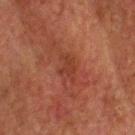<tbp_lesion>
  <biopsy_status>not biopsied; imaged during a skin examination</biopsy_status>
  <lighting>cross-polarized</lighting>
  <patient>
    <sex>male</sex>
    <age_approx>60</age_approx>
  </patient>
  <lesion_size>
    <long_diameter_mm_approx>3.5</long_diameter_mm_approx>
  </lesion_size>
  <automated_metrics>
    <area_mm2_approx>4.5</area_mm2_approx>
    <vs_skin_darker_L>5.0</vs_skin_darker_L>
    <vs_skin_contrast_norm>5.5</vs_skin_contrast_norm>
    <border_irregularity_0_10>5.5</border_irregularity_0_10>
    <color_variation_0_10>1.0</color_variation_0_10>
    <peripheral_color_asymmetry>0.5</peripheral_color_asymmetry>
    <nevus_likeness_0_100>0</nevus_likeness_0_100>
    <lesion_detection_confidence_0_100>100</lesion_detection_confidence_0_100>
  </automated_metrics>
  <image>
    <source>total-body photography crop</source>
    <field_of_view_mm>15</field_of_view_mm>
  </image>
  <site>head or neck</site>
</tbp_lesion>